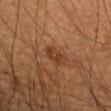Clinical impression:
No biopsy was performed on this lesion — it was imaged during a full skin examination and was not determined to be concerning.
Acquisition and patient details:
The lesion is located on the left upper arm. A roughly 15 mm field-of-view crop from a total-body skin photograph. Captured under cross-polarized illumination. A male patient aged around 55.